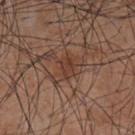Imaged during a routine full-body skin examination; the lesion was not biopsied and no histopathology is available.
Captured under white-light illumination.
Cropped from a whole-body photographic skin survey; the tile spans about 15 mm.
A male subject aged 53–57.
The total-body-photography lesion software estimated a footprint of about 4 mm², a shape eccentricity near 0.75, and two-axis asymmetry of about 0.25. The analysis additionally found an average lesion color of about L≈38 a*≈21 b*≈26 (CIELAB), a lesion–skin lightness drop of about 7, and a normalized border contrast of about 6.5.
The lesion is on the abdomen.
Approximately 2.5 mm at its widest.